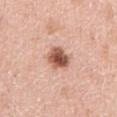workup = total-body-photography surveillance lesion; no biopsy
diameter = ≈3 mm
patient = female, aged 48 to 52
lighting = white-light
acquisition = ~15 mm crop, total-body skin-cancer survey
site = the right upper arm